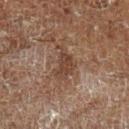follow-up = imaged on a skin check; not biopsied
tile lighting = cross-polarized illumination
acquisition = ~15 mm tile from a whole-body skin photo
subject = male, aged around 60
location = the left lower leg